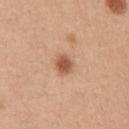Part of a total-body skin-imaging series; this lesion was reviewed on a skin check and was not flagged for biopsy.
On the chest.
Longest diameter approximately 2.5 mm.
This is a white-light tile.
A female subject, in their 30s.
A 15 mm close-up extracted from a 3D total-body photography capture.